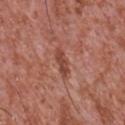Clinical impression:
Imaged during a routine full-body skin examination; the lesion was not biopsied and no histopathology is available.
Context:
The subject is a male aged around 45. A 15 mm crop from a total-body photograph taken for skin-cancer surveillance. On the chest. Approximately 3.5 mm at its widest. The tile uses white-light illumination. An algorithmic analysis of the crop reported a shape eccentricity near 0.95 and a shape-asymmetry score of about 0.3 (0 = symmetric). The analysis additionally found a lesion–skin lightness drop of about 10 and a lesion-to-skin contrast of about 7.5 (normalized; higher = more distinct). The software also gave a border-irregularity index near 3.5/10 and a within-lesion color-variation index near 0/10.